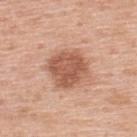On the upper back. Approximately 4.5 mm at its widest. A male patient, aged 58–62. This is a white-light tile. A 15 mm close-up tile from a total-body photography series done for melanoma screening.